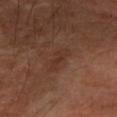The lesion was photographed on a routine skin check and not biopsied; there is no pathology result.
A male patient, aged around 50.
A 15 mm close-up tile from a total-body photography series done for melanoma screening.
From the left forearm.